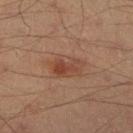Impression:
Imaged during a routine full-body skin examination; the lesion was not biopsied and no histopathology is available.
Acquisition and patient details:
Located on the leg. A 15 mm crop from a total-body photograph taken for skin-cancer surveillance. Longest diameter approximately 4.5 mm. A male patient in their 40s.